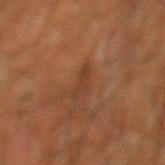{
  "biopsy_status": "not biopsied; imaged during a skin examination",
  "site": "mid back",
  "image": {
    "source": "total-body photography crop",
    "field_of_view_mm": 15
  },
  "lighting": "cross-polarized",
  "patient": {
    "sex": "male",
    "age_approx": 80
  },
  "automated_metrics": {
    "area_mm2_approx": 3.5,
    "eccentricity": 0.9,
    "shape_asymmetry": 0.45,
    "color_variation_0_10": 0.0,
    "peripheral_color_asymmetry": 0.0,
    "nevus_likeness_0_100": 5,
    "lesion_detection_confidence_0_100": 95
  },
  "lesion_size": {
    "long_diameter_mm_approx": 3.0
  }
}